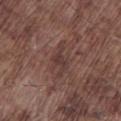– biopsy status · no biopsy performed (imaged during a skin exam)
– automated lesion analysis · a border-irregularity index near 7/10, a within-lesion color-variation index near 2/10, and a peripheral color-asymmetry measure near 0.5; a lesion-detection confidence of about 55/100
– subject · male, approximately 75 years of age
– acquisition · ~15 mm tile from a whole-body skin photo
– site · the left lower leg